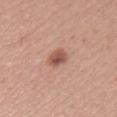{
  "biopsy_status": "not biopsied; imaged during a skin examination",
  "patient": {
    "sex": "female",
    "age_approx": 25
  },
  "automated_metrics": {
    "area_mm2_approx": 4.5,
    "eccentricity": 0.75,
    "shape_asymmetry": 0.25,
    "border_irregularity_0_10": 2.5,
    "color_variation_0_10": 5.0,
    "peripheral_color_asymmetry": 2.0
  },
  "site": "left upper arm",
  "lighting": "white-light",
  "image": {
    "source": "total-body photography crop",
    "field_of_view_mm": 15
  }
}